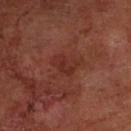Notes:
* notes: catalogued during a skin exam; not biopsied
* lesion size: ≈3 mm
* TBP lesion metrics: an area of roughly 5 mm², an outline eccentricity of about 0.75 (0 = round, 1 = elongated), and two-axis asymmetry of about 0.35; a within-lesion color-variation index near 2/10 and a peripheral color-asymmetry measure near 0.5; an automated nevus-likeness rating near 0 out of 100 and a lesion-detection confidence of about 100/100
* site: the left forearm
* patient: male, in their mid- to late 60s
* illumination: cross-polarized illumination
* image source: 15 mm crop, total-body photography